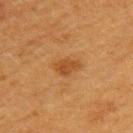workup: no biopsy performed (imaged during a skin exam)
imaging modality: total-body-photography crop, ~15 mm field of view
tile lighting: cross-polarized
site: the left upper arm
patient: female, aged around 40
lesion diameter: ~3 mm (longest diameter)